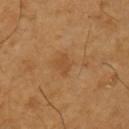Findings:
– workup: total-body-photography surveillance lesion; no biopsy
– subject: male, aged around 65
– acquisition: total-body-photography crop, ~15 mm field of view
– automated lesion analysis: an area of roughly 2.5 mm² and two-axis asymmetry of about 0.5; border irregularity of about 5 on a 0–10 scale, internal color variation of about 0 on a 0–10 scale, and a peripheral color-asymmetry measure near 0; lesion-presence confidence of about 100/100
– site: the left upper arm
– tile lighting: cross-polarized